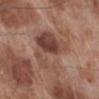Q: Was this lesion biopsied?
A: imaged on a skin check; not biopsied
Q: What is the lesion's diameter?
A: ~6 mm (longest diameter)
Q: What kind of image is this?
A: total-body-photography crop, ~15 mm field of view
Q: Illumination type?
A: white-light
Q: Where on the body is the lesion?
A: the right lower leg
Q: What did automated image analysis measure?
A: a border-irregularity rating of about 7/10 and a color-variation rating of about 7/10; a classifier nevus-likeness of about 0/100 and a detector confidence of about 100 out of 100 that the crop contains a lesion
Q: What are the patient's age and sex?
A: male, aged around 70